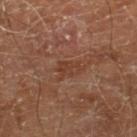notes — total-body-photography surveillance lesion; no biopsy | image source — 15 mm crop, total-body photography | image-analysis metrics — a lesion area of about 4 mm², an eccentricity of roughly 0.75, and a shape-asymmetry score of about 0.35 (0 = symmetric); a lesion color around L≈38 a*≈21 b*≈29 in CIELAB; a border-irregularity rating of about 3.5/10 and a color-variation rating of about 1.5/10 | patient — male, aged 68–72 | site — the right lower leg | lesion diameter — ~2.5 mm (longest diameter) | tile lighting — cross-polarized.